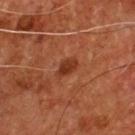Part of a total-body skin-imaging series; this lesion was reviewed on a skin check and was not flagged for biopsy. The subject is a male aged around 55. This is a cross-polarized tile. The lesion is located on the chest. A close-up tile cropped from a whole-body skin photograph, about 15 mm across.